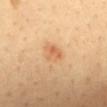Clinical impression: No biopsy was performed on this lesion — it was imaged during a full skin examination and was not determined to be concerning. Background: Longest diameter approximately 2.5 mm. A female patient aged around 50. The lesion is on the mid back. Imaged with cross-polarized lighting. A 15 mm crop from a total-body photograph taken for skin-cancer surveillance.